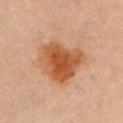{"biopsy_status": "not biopsied; imaged during a skin examination", "automated_metrics": {"cielab_L": 46, "cielab_a": 23, "cielab_b": 34, "nevus_likeness_0_100": 100, "lesion_detection_confidence_0_100": 100}, "patient": {"sex": "female", "age_approx": 45}, "site": "left upper arm", "image": {"source": "total-body photography crop", "field_of_view_mm": 15}, "lighting": "cross-polarized"}